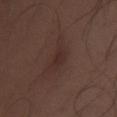| feature | finding |
|---|---|
| notes | imaged on a skin check; not biopsied |
| diameter | about 2.5 mm |
| patient | male, approximately 30 years of age |
| image | total-body-photography crop, ~15 mm field of view |
| image-analysis metrics | an average lesion color of about L≈28 a*≈17 b*≈18 (CIELAB), roughly 5 lightness units darker than nearby skin, and a normalized lesion–skin contrast near 5.5; border irregularity of about 3 on a 0–10 scale, internal color variation of about 1 on a 0–10 scale, and peripheral color asymmetry of about 0.5 |
| location | the chest |
| lighting | white-light illumination |